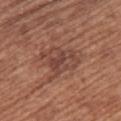{
  "biopsy_status": "not biopsied; imaged during a skin examination",
  "automated_metrics": {
    "cielab_L": 44,
    "cielab_a": 22,
    "cielab_b": 26,
    "vs_skin_darker_L": 8.0,
    "vs_skin_contrast_norm": 6.5
  },
  "lighting": "white-light",
  "site": "back",
  "image": {
    "source": "total-body photography crop",
    "field_of_view_mm": 15
  },
  "lesion_size": {
    "long_diameter_mm_approx": 5.0
  },
  "patient": {
    "sex": "female",
    "age_approx": 60
  }
}